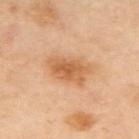Q: What are the patient's age and sex?
A: female, aged approximately 45
Q: Illumination type?
A: cross-polarized illumination
Q: How was this image acquired?
A: ~15 mm tile from a whole-body skin photo
Q: What is the anatomic site?
A: the upper back
Q: Automated lesion metrics?
A: an average lesion color of about L≈62 a*≈23 b*≈40 (CIELAB), roughly 10 lightness units darker than nearby skin, and a normalized border contrast of about 7.5; a border-irregularity rating of about 3/10 and a color-variation rating of about 4/10